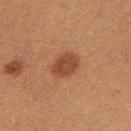Assessment: The lesion was photographed on a routine skin check and not biopsied; there is no pathology result. Clinical summary: Longest diameter approximately 3.5 mm. A female subject, aged 23–27. A lesion tile, about 15 mm wide, cut from a 3D total-body photograph. The total-body-photography lesion software estimated a footprint of about 7.5 mm², an outline eccentricity of about 0.7 (0 = round, 1 = elongated), and a shape-asymmetry score of about 0.2 (0 = symmetric). The analysis additionally found a border-irregularity rating of about 2/10, a within-lesion color-variation index near 2.5/10, and radial color variation of about 1. The analysis additionally found a classifier nevus-likeness of about 95/100 and lesion-presence confidence of about 100/100. The lesion is located on the arm. The tile uses white-light illumination.